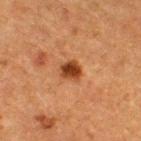An algorithmic analysis of the crop reported a mean CIELAB color near L≈34 a*≈23 b*≈33, roughly 13 lightness units darker than nearby skin, and a normalized lesion–skin contrast near 11. It also reported a color-variation rating of about 3/10. It also reported a nevus-likeness score of about 100/100 and a lesion-detection confidence of about 100/100.
This image is a 15 mm lesion crop taken from a total-body photograph.
This is a cross-polarized tile.
A male patient, approximately 50 years of age.
The lesion is located on the upper back.